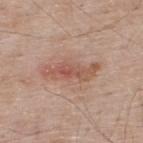Q: Was a biopsy performed?
A: total-body-photography surveillance lesion; no biopsy
Q: Illumination type?
A: white-light illumination
Q: How was this image acquired?
A: 15 mm crop, total-body photography
Q: Lesion location?
A: the upper back
Q: Patient demographics?
A: male, approximately 65 years of age